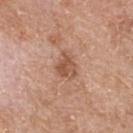| field | value |
|---|---|
| workup | total-body-photography surveillance lesion; no biopsy |
| patient | female, aged 73–77 |
| lesion size | ~3.5 mm (longest diameter) |
| acquisition | total-body-photography crop, ~15 mm field of view |
| anatomic site | the upper back |